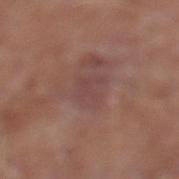Part of a total-body skin-imaging series; this lesion was reviewed on a skin check and was not flagged for biopsy. The subject is a male aged around 65. On the leg. A 15 mm close-up extracted from a 3D total-body photography capture.